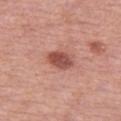Clinical impression:
No biopsy was performed on this lesion — it was imaged during a full skin examination and was not determined to be concerning.
Acquisition and patient details:
A female patient in their mid-50s. The lesion is on the left thigh. This is a white-light tile. A 15 mm close-up extracted from a 3D total-body photography capture.